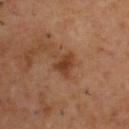Clinical impression:
The lesion was tiled from a total-body skin photograph and was not biopsied.
Image and clinical context:
A male subject approximately 55 years of age. On the back. A lesion tile, about 15 mm wide, cut from a 3D total-body photograph.